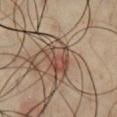Findings:
- biopsy status: no biopsy performed (imaged during a skin exam)
- diameter: ~4.5 mm (longest diameter)
- tile lighting: cross-polarized illumination
- location: the chest
- patient: male, approximately 65 years of age
- image source: total-body-photography crop, ~15 mm field of view